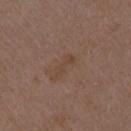The lesion was photographed on a routine skin check and not biopsied; there is no pathology result. A male subject aged around 50. Automated image analysis of the tile measured a lesion area of about 3.5 mm² and two-axis asymmetry of about 0.3. The software also gave a lesion color around L≈42 a*≈17 b*≈26 in CIELAB. The analysis additionally found a nevus-likeness score of about 0/100. The lesion is located on the right upper arm. A close-up tile cropped from a whole-body skin photograph, about 15 mm across.